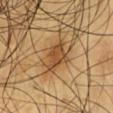lesion size — about 3.5 mm; site — the chest; imaging modality — ~15 mm crop, total-body skin-cancer survey; patient — male, roughly 60 years of age; tile lighting — cross-polarized illumination.